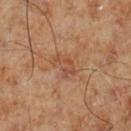  site: left lower leg
  lighting: cross-polarized
  patient:
    sex: male
    age_approx: 70
  automated_metrics:
    area_mm2_approx: 5.0
    eccentricity: 0.8
    border_irregularity_0_10: 5.0
    color_variation_0_10: 2.5
    peripheral_color_asymmetry: 1.0
    nevus_likeness_0_100: 0
    lesion_detection_confidence_0_100: 100
  image:
    source: total-body photography crop
    field_of_view_mm: 15